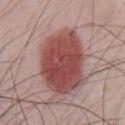biopsy status = catalogued during a skin exam; not biopsied
body site = the chest
lesion size = ≈8 mm
illumination = white-light illumination
subject = male, about 40 years old
automated metrics = a border-irregularity rating of about 1.5/10 and a peripheral color-asymmetry measure near 1.5; a nevus-likeness score of about 100/100 and lesion-presence confidence of about 100/100
image = 15 mm crop, total-body photography